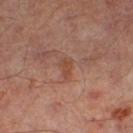{
  "biopsy_status": "not biopsied; imaged during a skin examination",
  "site": "left thigh",
  "automated_metrics": {
    "area_mm2_approx": 3.0,
    "eccentricity": 0.85,
    "shape_asymmetry": 0.35,
    "cielab_L": 44,
    "cielab_a": 22,
    "cielab_b": 29,
    "vs_skin_darker_L": 6.0
  },
  "image": {
    "source": "total-body photography crop",
    "field_of_view_mm": 15
  },
  "patient": {
    "sex": "male",
    "age_approx": 65
  },
  "lighting": "cross-polarized"
}